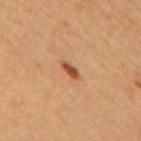• follow-up · total-body-photography surveillance lesion; no biopsy
• patient · female
• lesion diameter · ~2.5 mm (longest diameter)
• anatomic site · the upper back
• imaging modality · total-body-photography crop, ~15 mm field of view